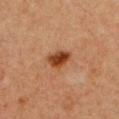biopsy status: no biopsy performed (imaged during a skin exam)
image source: ~15 mm crop, total-body skin-cancer survey
illumination: cross-polarized illumination
subject: female, aged 38 to 42
body site: the front of the torso